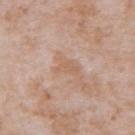{"site": "abdomen", "lesion_size": {"long_diameter_mm_approx": 3.0}, "image": {"source": "total-body photography crop", "field_of_view_mm": 15}, "lighting": "white-light", "patient": {"sex": "male", "age_approx": 65}}